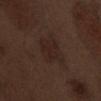Case summary:
* notes: total-body-photography surveillance lesion; no biopsy
* automated metrics: an area of roughly 10 mm² and a shape-asymmetry score of about 0.25 (0 = symmetric); border irregularity of about 3.5 on a 0–10 scale, a within-lesion color-variation index near 2.5/10, and peripheral color asymmetry of about 1; a classifier nevus-likeness of about 5/100
* imaging modality: total-body-photography crop, ~15 mm field of view
* lesion diameter: ≈4.5 mm
* lighting: white-light
* anatomic site: the abdomen
* subject: male, in their 70s